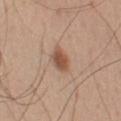Recorded during total-body skin imaging; not selected for excision or biopsy.
A lesion tile, about 15 mm wide, cut from a 3D total-body photograph.
About 3 mm across.
The total-body-photography lesion software estimated an area of roughly 5 mm², an outline eccentricity of about 0.75 (0 = round, 1 = elongated), and a symmetry-axis asymmetry near 0.3. The software also gave a mean CIELAB color near L≈52 a*≈21 b*≈30 and a lesion–skin lightness drop of about 12. The analysis additionally found lesion-presence confidence of about 100/100.
From the left upper arm.
The patient is a male in their mid- to late 50s.
This is a white-light tile.